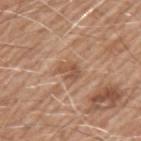Captured during whole-body skin photography for melanoma surveillance; the lesion was not biopsied. The subject is a male aged 58–62. This is a white-light tile. A roughly 15 mm field-of-view crop from a total-body skin photograph. Located on the left upper arm. Approximately 3 mm at its widest.